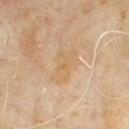notes=catalogued during a skin exam; not biopsied
subject=male, approximately 60 years of age
body site=the mid back
imaging modality=total-body-photography crop, ~15 mm field of view